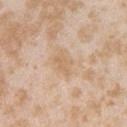tile lighting — white-light | site — the arm | lesion diameter — ~3 mm (longest diameter) | acquisition — ~15 mm tile from a whole-body skin photo | subject — female, aged approximately 25.